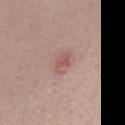Recorded during total-body skin imaging; not selected for excision or biopsy.
The subject is a female aged approximately 35.
The lesion's longest dimension is about 2.5 mm.
Imaged with white-light lighting.
The lesion is located on the right upper arm.
A 15 mm crop from a total-body photograph taken for skin-cancer surveillance.